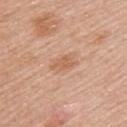{
  "biopsy_status": "not biopsied; imaged during a skin examination",
  "image": {
    "source": "total-body photography crop",
    "field_of_view_mm": 15
  },
  "site": "left upper arm",
  "lighting": "white-light",
  "patient": {
    "sex": "female",
    "age_approx": 50
  }
}